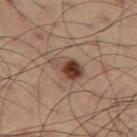Q: Was this lesion biopsied?
A: catalogued during a skin exam; not biopsied
Q: Who is the patient?
A: male, roughly 60 years of age
Q: How was the tile lit?
A: cross-polarized
Q: What did automated image analysis measure?
A: a lesion color around L≈36 a*≈16 b*≈23 in CIELAB and a normalized border contrast of about 10; a border-irregularity index near 3/10; a nevus-likeness score of about 95/100 and a lesion-detection confidence of about 100/100
Q: Lesion size?
A: ~4 mm (longest diameter)
Q: What is the imaging modality?
A: ~15 mm crop, total-body skin-cancer survey